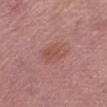| key | value |
|---|---|
| follow-up | catalogued during a skin exam; not biopsied |
| image | ~15 mm tile from a whole-body skin photo |
| tile lighting | white-light illumination |
| automated lesion analysis | internal color variation of about 2.5 on a 0–10 scale and a peripheral color-asymmetry measure near 1; a classifier nevus-likeness of about 10/100 and lesion-presence confidence of about 100/100 |
| location | the right thigh |
| lesion diameter | ~3.5 mm (longest diameter) |
| patient | female, in their 60s |